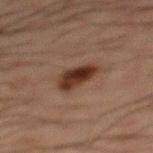Q: Is there a histopathology result?
A: catalogued during a skin exam; not biopsied
Q: What are the patient's age and sex?
A: male, roughly 50 years of age
Q: How large is the lesion?
A: ≈4.5 mm
Q: What lighting was used for the tile?
A: cross-polarized illumination
Q: Automated lesion metrics?
A: an area of roughly 8.5 mm² and an eccentricity of roughly 0.85; about 11 CIELAB-L* units darker than the surrounding skin and a lesion-to-skin contrast of about 11.5 (normalized; higher = more distinct); a classifier nevus-likeness of about 95/100 and lesion-presence confidence of about 100/100
Q: Lesion location?
A: the mid back
Q: What kind of image is this?
A: ~15 mm crop, total-body skin-cancer survey